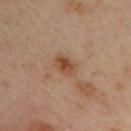| key | value |
|---|---|
| follow-up | total-body-photography surveillance lesion; no biopsy |
| diameter | about 2.5 mm |
| lighting | cross-polarized illumination |
| automated lesion analysis | an eccentricity of roughly 0.75 and a symmetry-axis asymmetry near 0.2 |
| site | the upper back |
| subject | female, aged approximately 40 |
| image | ~15 mm tile from a whole-body skin photo |